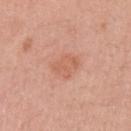follow-up — no biopsy performed (imaged during a skin exam) | automated metrics — a footprint of about 6 mm², an eccentricity of roughly 0.4, and a shape-asymmetry score of about 0.2 (0 = symmetric); an average lesion color of about L≈61 a*≈25 b*≈32 (CIELAB), roughly 7 lightness units darker than nearby skin, and a normalized border contrast of about 5 | lighting — white-light | lesion size — ≈2.5 mm | image source — 15 mm crop, total-body photography | patient — female, roughly 55 years of age | anatomic site — the arm.